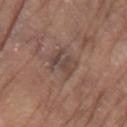Clinical impression: The lesion was tiled from a total-body skin photograph and was not biopsied. Acquisition and patient details: A male subject about 80 years old. A 15 mm close-up tile from a total-body photography series done for melanoma screening. The lesion is located on the arm.